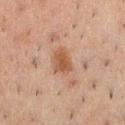The lesion was tiled from a total-body skin photograph and was not biopsied. On the chest. Automated tile analysis of the lesion measured an eccentricity of roughly 0.75 and a shape-asymmetry score of about 0.2 (0 = symmetric). The analysis additionally found a border-irregularity rating of about 2/10, a within-lesion color-variation index near 3.5/10, and peripheral color asymmetry of about 1. This is a cross-polarized tile. Approximately 3.5 mm at its widest. Cropped from a whole-body photographic skin survey; the tile spans about 15 mm. The subject is a male aged 48 to 52.